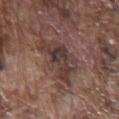Q: Was this lesion biopsied?
A: imaged on a skin check; not biopsied
Q: What kind of image is this?
A: ~15 mm tile from a whole-body skin photo
Q: Patient demographics?
A: male, aged approximately 75
Q: What is the anatomic site?
A: the chest